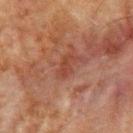Imaged during a routine full-body skin examination; the lesion was not biopsied and no histopathology is available.
A close-up tile cropped from a whole-body skin photograph, about 15 mm across.
The patient is a male aged 63 to 67.
The recorded lesion diameter is about 3 mm.
Captured under cross-polarized illumination.
The lesion is located on the upper back.